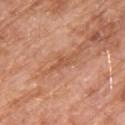notes — imaged on a skin check; not biopsied
image — 15 mm crop, total-body photography
patient — male, aged approximately 80
location — the upper back